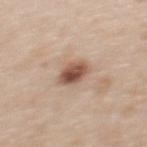Assessment:
Recorded during total-body skin imaging; not selected for excision or biopsy.
Background:
The subject is a female aged approximately 50. A close-up tile cropped from a whole-body skin photograph, about 15 mm across. Located on the upper back. The total-body-photography lesion software estimated a shape eccentricity near 0.75 and two-axis asymmetry of about 0.15. And it measured a lesion color around L≈53 a*≈20 b*≈27 in CIELAB, a lesion–skin lightness drop of about 16, and a normalized lesion–skin contrast near 10.5. And it measured border irregularity of about 1.5 on a 0–10 scale and a color-variation rating of about 5.5/10. The recorded lesion diameter is about 3.5 mm. Imaged with white-light lighting.